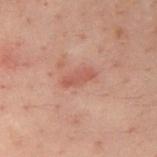Clinical impression:
Captured during whole-body skin photography for melanoma surveillance; the lesion was not biopsied.
Acquisition and patient details:
A male subject aged around 40. The tile uses cross-polarized illumination. On the right upper arm. Longest diameter approximately 3.5 mm. A region of skin cropped from a whole-body photographic capture, roughly 15 mm wide. Automated tile analysis of the lesion measured a within-lesion color-variation index near 1/10.